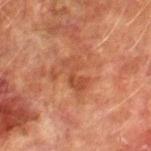The lesion was photographed on a routine skin check and not biopsied; there is no pathology result.
This image is a 15 mm lesion crop taken from a total-body photograph.
Located on the leg.
Automated image analysis of the tile measured a lesion color around L≈41 a*≈24 b*≈31 in CIELAB, about 7 CIELAB-L* units darker than the surrounding skin, and a lesion-to-skin contrast of about 5.5 (normalized; higher = more distinct). The analysis additionally found border irregularity of about 5.5 on a 0–10 scale, a color-variation rating of about 3/10, and a peripheral color-asymmetry measure near 1.
Longest diameter approximately 3.5 mm.
The patient is a male aged around 75.